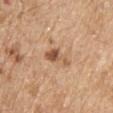No biopsy was performed on this lesion — it was imaged during a full skin examination and was not determined to be concerning. Imaged with white-light lighting. The lesion is located on the upper back. A male subject, roughly 70 years of age. Measured at roughly 3.5 mm in maximum diameter. Cropped from a whole-body photographic skin survey; the tile spans about 15 mm.